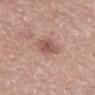{
  "patient": {
    "sex": "male",
    "age_approx": 80
  },
  "lesion_size": {
    "long_diameter_mm_approx": 4.5
  },
  "image": {
    "source": "total-body photography crop",
    "field_of_view_mm": 15
  },
  "automated_metrics": {
    "area_mm2_approx": 6.0,
    "eccentricity": 0.9,
    "shape_asymmetry": 0.3,
    "vs_skin_darker_L": 11.0,
    "border_irregularity_0_10": 3.5,
    "peripheral_color_asymmetry": 1.0,
    "nevus_likeness_0_100": 50
  },
  "lighting": "white-light",
  "site": "mid back"
}